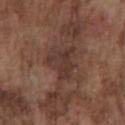Assessment:
Imaged during a routine full-body skin examination; the lesion was not biopsied and no histopathology is available.
Image and clinical context:
A lesion tile, about 15 mm wide, cut from a 3D total-body photograph. The subject is a male aged 73 to 77. The lesion is located on the front of the torso. The total-body-photography lesion software estimated a border-irregularity index near 6/10, a color-variation rating of about 2.5/10, and a peripheral color-asymmetry measure near 1. The software also gave an automated nevus-likeness rating near 0 out of 100 and a lesion-detection confidence of about 95/100.